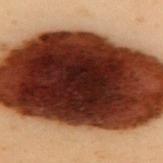Case summary:
• biopsy status — total-body-photography surveillance lesion; no biopsy
• acquisition — ~15 mm crop, total-body skin-cancer survey
• patient — female, in their 60s
• automated metrics — a lesion color around L≈25 a*≈25 b*≈27 in CIELAB and about 35 CIELAB-L* units darker than the surrounding skin
• lighting — cross-polarized illumination
• anatomic site — the upper back
• size — about 17.5 mm